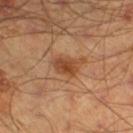Recorded during total-body skin imaging; not selected for excision or biopsy.
Located on the left lower leg.
A male patient roughly 45 years of age.
Approximately 3 mm at its widest.
This is a cross-polarized tile.
This image is a 15 mm lesion crop taken from a total-body photograph.